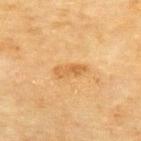Findings:
- anatomic site — the upper back
- patient — female, in their 80s
- image source — total-body-photography crop, ~15 mm field of view